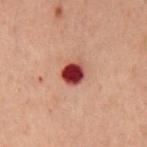Findings:
• acquisition — ~15 mm tile from a whole-body skin photo
• patient — male, aged approximately 60
• site — the chest